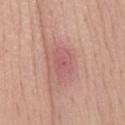biopsy status: total-body-photography surveillance lesion; no biopsy | site: the back | illumination: white-light illumination | image source: ~15 mm crop, total-body skin-cancer survey | lesion diameter: ≈3.5 mm | automated metrics: a lesion–skin lightness drop of about 7 and a normalized lesion–skin contrast near 5 | subject: female, roughly 65 years of age.